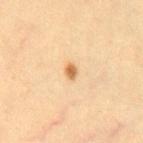{
  "biopsy_status": "not biopsied; imaged during a skin examination",
  "lighting": "cross-polarized",
  "image": {
    "source": "total-body photography crop",
    "field_of_view_mm": 15
  },
  "patient": {
    "sex": "female",
    "age_approx": 30
  },
  "site": "front of the torso",
  "lesion_size": {
    "long_diameter_mm_approx": 2.0
  }
}